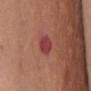No biopsy was performed on this lesion — it was imaged during a full skin examination and was not determined to be concerning. The lesion's longest dimension is about 3 mm. A female patient, aged around 55. A region of skin cropped from a whole-body photographic capture, roughly 15 mm wide. Located on the chest. An algorithmic analysis of the crop reported an area of roughly 5 mm², an outline eccentricity of about 0.6 (0 = round, 1 = elongated), and two-axis asymmetry of about 0.2. It also reported roughly 10 lightness units darker than nearby skin. It also reported a nevus-likeness score of about 0/100 and a detector confidence of about 100 out of 100 that the crop contains a lesion. Imaged with white-light lighting.